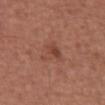Findings:
- subject: male, in their mid-60s
- acquisition: 15 mm crop, total-body photography
- illumination: white-light illumination
- anatomic site: the abdomen